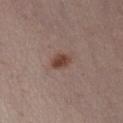Captured during whole-body skin photography for melanoma surveillance; the lesion was not biopsied. Measured at roughly 2.5 mm in maximum diameter. The lesion is on the abdomen. A 15 mm close-up extracted from a 3D total-body photography capture. The total-body-photography lesion software estimated an outline eccentricity of about 0.7 (0 = round, 1 = elongated) and a symmetry-axis asymmetry near 0.3. And it measured a border-irregularity index near 2.5/10, a color-variation rating of about 3/10, and peripheral color asymmetry of about 1. The patient is a female roughly 30 years of age.